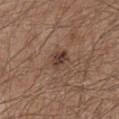biopsy_status: not biopsied; imaged during a skin examination
lighting: white-light
automated_metrics:
  area_mm2_approx: 4.0
  eccentricity: 0.65
  shape_asymmetry: 0.25
  cielab_L: 39
  cielab_a: 17
  cielab_b: 25
  vs_skin_darker_L: 10.0
  vs_skin_contrast_norm: 8.5
  nevus_likeness_0_100: 40
  lesion_detection_confidence_0_100: 100
patient:
  sex: male
  age_approx: 65
site: front of the torso
image:
  source: total-body photography crop
  field_of_view_mm: 15
lesion_size:
  long_diameter_mm_approx: 2.5